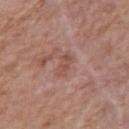Impression: Recorded during total-body skin imaging; not selected for excision or biopsy. Context: Measured at roughly 3.5 mm in maximum diameter. From the right upper arm. A female patient about 75 years old. Cropped from a total-body skin-imaging series; the visible field is about 15 mm. This is a white-light tile.